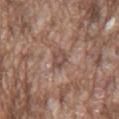biopsy status: catalogued during a skin exam; not biopsied | image-analysis metrics: an outline eccentricity of about 0.85 (0 = round, 1 = elongated) and a symmetry-axis asymmetry near 0.3; a lesion color around L≈48 a*≈18 b*≈24 in CIELAB and roughly 8 lightness units darker than nearby skin; a nevus-likeness score of about 0/100 and lesion-presence confidence of about 55/100 | anatomic site: the chest | lesion size: about 2.5 mm | image source: total-body-photography crop, ~15 mm field of view | patient: male, aged approximately 75.